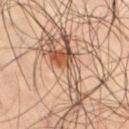Recorded during total-body skin imaging; not selected for excision or biopsy.
The patient is a male roughly 60 years of age.
Captured under cross-polarized illumination.
The lesion is on the leg.
A 15 mm crop from a total-body photograph taken for skin-cancer surveillance.